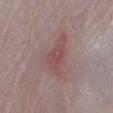Findings:
– biopsy status · no biopsy performed (imaged during a skin exam)
– patient · male, roughly 65 years of age
– location · the right lower leg
– illumination · white-light
– image source · total-body-photography crop, ~15 mm field of view